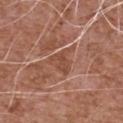biopsy_status: not biopsied; imaged during a skin examination
lesion_size:
  long_diameter_mm_approx: 3.5
site: front of the torso
patient:
  sex: male
  age_approx: 75
image:
  source: total-body photography crop
  field_of_view_mm: 15